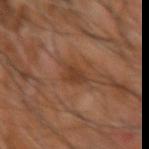Part of a total-body skin-imaging series; this lesion was reviewed on a skin check and was not flagged for biopsy. Imaged with cross-polarized lighting. About 3.5 mm across. The lesion is located on the arm. A male subject in their mid- to late 60s. This image is a 15 mm lesion crop taken from a total-body photograph.